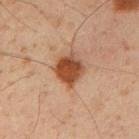Clinical summary:
Measured at roughly 3.5 mm in maximum diameter. A male subject about 50 years old. Automated tile analysis of the lesion measured an eccentricity of roughly 0.45 and a shape-asymmetry score of about 0.2 (0 = symmetric). The analysis additionally found an average lesion color of about L≈43 a*≈22 b*≈31 (CIELAB), roughly 14 lightness units darker than nearby skin, and a normalized lesion–skin contrast near 11. The software also gave a border-irregularity index near 2/10, internal color variation of about 5 on a 0–10 scale, and a peripheral color-asymmetry measure near 1.5. The software also gave an automated nevus-likeness rating near 100 out of 100 and a detector confidence of about 100 out of 100 that the crop contains a lesion. A 15 mm close-up tile from a total-body photography series done for melanoma screening. The lesion is on the right upper arm.